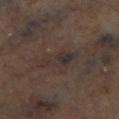Q: Was a biopsy performed?
A: catalogued during a skin exam; not biopsied
Q: Lesion size?
A: about 4.5 mm
Q: Lesion location?
A: the right lower leg
Q: What lighting was used for the tile?
A: cross-polarized
Q: What is the imaging modality?
A: total-body-photography crop, ~15 mm field of view